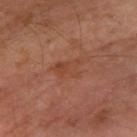This lesion was catalogued during total-body skin photography and was not selected for biopsy. The subject is a male approximately 60 years of age. The lesion is located on the left forearm. The total-body-photography lesion software estimated roughly 5 lightness units darker than nearby skin. The software also gave a border-irregularity index near 5.5/10 and radial color variation of about 1. Longest diameter approximately 3.5 mm. A lesion tile, about 15 mm wide, cut from a 3D total-body photograph. Imaged with cross-polarized lighting.